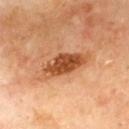Recorded during total-body skin imaging; not selected for excision or biopsy. A close-up tile cropped from a whole-body skin photograph, about 15 mm across. A male subject, approximately 70 years of age. The lesion is on the mid back.